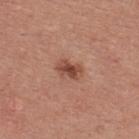| feature | finding |
|---|---|
| follow-up | total-body-photography surveillance lesion; no biopsy |
| patient | male, aged approximately 40 |
| site | the back |
| acquisition | 15 mm crop, total-body photography |
| TBP lesion metrics | about 11 CIELAB-L* units darker than the surrounding skin and a normalized border contrast of about 8.5; a border-irregularity index near 2.5/10 and peripheral color asymmetry of about 1 |
| lesion diameter | ~3 mm (longest diameter) |
| tile lighting | white-light illumination |